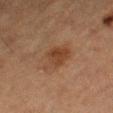notes: total-body-photography surveillance lesion; no biopsy
subject: male, in their mid- to late 70s
body site: the right thigh
lighting: cross-polarized illumination
lesion diameter: about 4 mm
imaging modality: ~15 mm crop, total-body skin-cancer survey